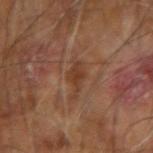biopsy_status: not biopsied; imaged during a skin examination